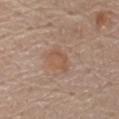This lesion was catalogued during total-body skin photography and was not selected for biopsy. A region of skin cropped from a whole-body photographic capture, roughly 15 mm wide. A male patient aged 68 to 72. The lesion is located on the front of the torso. Longest diameter approximately 3 mm.